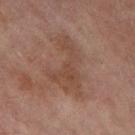Part of a total-body skin-imaging series; this lesion was reviewed on a skin check and was not flagged for biopsy.
A female subject aged approximately 80.
Cropped from a whole-body photographic skin survey; the tile spans about 15 mm.
The lesion is located on the leg.
The lesion's longest dimension is about 7.5 mm.